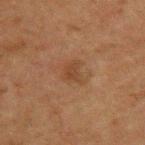{"biopsy_status": "not biopsied; imaged during a skin examination", "automated_metrics": {"eccentricity": 0.55, "shape_asymmetry": 0.3, "border_irregularity_0_10": 3.0, "peripheral_color_asymmetry": 0.5, "lesion_detection_confidence_0_100": 100}, "lighting": "cross-polarized", "image": {"source": "total-body photography crop", "field_of_view_mm": 15}, "lesion_size": {"long_diameter_mm_approx": 2.5}, "site": "upper back", "patient": {"sex": "male", "age_approx": 60}}